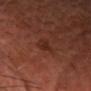  site: head or neck
  automated_metrics:
    eccentricity: 0.8
    cielab_L: 29
    cielab_a: 24
    cielab_b: 27
    vs_skin_contrast_norm: 6.0
    border_irregularity_0_10: 4.0
    color_variation_0_10: 1.0
  lesion_size:
    long_diameter_mm_approx: 3.0
  image:
    source: total-body photography crop
    field_of_view_mm: 15
  patient:
    sex: female
    age_approx: 55
  lighting: cross-polarized